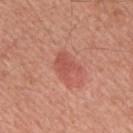workup — catalogued during a skin exam; not biopsied
body site — the mid back
patient — male, about 65 years old
image source — ~15 mm tile from a whole-body skin photo
size — about 3.5 mm
image-analysis metrics — a lesion area of about 6.5 mm², a shape eccentricity near 0.65, and a symmetry-axis asymmetry near 0.35